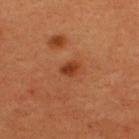Part of a total-body skin-imaging series; this lesion was reviewed on a skin check and was not flagged for biopsy. From the upper back. A male subject aged around 65. A 15 mm close-up extracted from a 3D total-body photography capture. The lesion-visualizer software estimated an area of roughly 3 mm², an eccentricity of roughly 0.7, and a symmetry-axis asymmetry near 0.3. And it measured a nevus-likeness score of about 85/100 and a lesion-detection confidence of about 100/100.